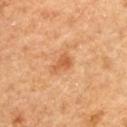Part of a total-body skin-imaging series; this lesion was reviewed on a skin check and was not flagged for biopsy. The lesion is on the upper back. The lesion-visualizer software estimated an area of roughly 3.5 mm², an outline eccentricity of about 0.75 (0 = round, 1 = elongated), and two-axis asymmetry of about 0.45. It also reported roughly 9 lightness units darker than nearby skin and a normalized lesion–skin contrast near 6.5. The analysis additionally found lesion-presence confidence of about 100/100. A female patient in their 60s. A region of skin cropped from a whole-body photographic capture, roughly 15 mm wide. The tile uses cross-polarized illumination.